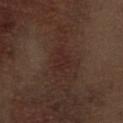biopsy status — catalogued during a skin exam; not biopsied | patient — male, in their 70s | imaging modality — total-body-photography crop, ~15 mm field of view | size — ≈3 mm | lighting — white-light | image-analysis metrics — an area of roughly 4.5 mm², an eccentricity of roughly 0.8, and two-axis asymmetry of about 0.4 | location — the front of the torso.